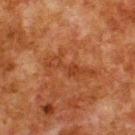Impression:
The lesion was photographed on a routine skin check and not biopsied; there is no pathology result.
Clinical summary:
A male subject aged approximately 80. A 15 mm close-up extracted from a 3D total-body photography capture. This is a cross-polarized tile. The lesion-visualizer software estimated a mean CIELAB color near L≈34 a*≈23 b*≈32, about 6 CIELAB-L* units darker than the surrounding skin, and a lesion-to-skin contrast of about 5.5 (normalized; higher = more distinct). The software also gave a border-irregularity rating of about 9/10 and peripheral color asymmetry of about 0.5. The software also gave a classifier nevus-likeness of about 0/100 and a lesion-detection confidence of about 100/100. The lesion is on the upper back.